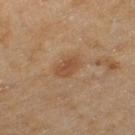Part of a total-body skin-imaging series; this lesion was reviewed on a skin check and was not flagged for biopsy. A 15 mm close-up tile from a total-body photography series done for melanoma screening. The lesion-visualizer software estimated an automated nevus-likeness rating near 65 out of 100 and a lesion-detection confidence of about 100/100. Located on the left thigh. A female subject, aged approximately 60. Captured under cross-polarized illumination.